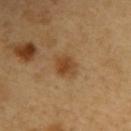{"biopsy_status": "not biopsied; imaged during a skin examination", "lighting": "cross-polarized", "lesion_size": {"long_diameter_mm_approx": 3.5}, "site": "right upper arm", "image": {"source": "total-body photography crop", "field_of_view_mm": 15}, "automated_metrics": {"nevus_likeness_0_100": 90, "lesion_detection_confidence_0_100": 100}, "patient": {"sex": "male", "age_approx": 60}}